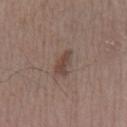On the left lower leg.
The tile uses white-light illumination.
Longest diameter approximately 3.5 mm.
A male patient, in their mid- to late 60s.
The lesion-visualizer software estimated an area of roughly 4.5 mm² and an eccentricity of roughly 0.9. The analysis additionally found a border-irregularity rating of about 3.5/10, a color-variation rating of about 2/10, and a peripheral color-asymmetry measure near 0.5. And it measured a nevus-likeness score of about 5/100 and a detector confidence of about 100 out of 100 that the crop contains a lesion.
A lesion tile, about 15 mm wide, cut from a 3D total-body photograph.